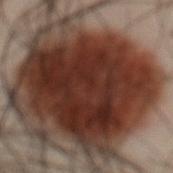Q: Is there a histopathology result?
A: catalogued during a skin exam; not biopsied
Q: What are the patient's age and sex?
A: male, aged 48–52
Q: What kind of image is this?
A: ~15 mm crop, total-body skin-cancer survey
Q: What is the anatomic site?
A: the front of the torso